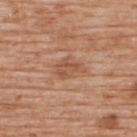notes: total-body-photography surveillance lesion; no biopsy
illumination: white-light illumination
anatomic site: the upper back
subject: male, aged 58–62
imaging modality: 15 mm crop, total-body photography
lesion diameter: ≈3.5 mm